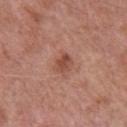Acquisition and patient details:
The lesion's longest dimension is about 2.5 mm. A 15 mm crop from a total-body photograph taken for skin-cancer surveillance. The patient is a male roughly 55 years of age. The tile uses white-light illumination. The lesion is on the arm.